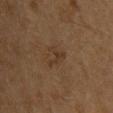Findings:
– biopsy status — catalogued during a skin exam; not biopsied
– imaging modality — ~15 mm tile from a whole-body skin photo
– subject — male, approximately 60 years of age